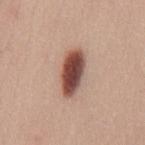No biopsy was performed on this lesion — it was imaged during a full skin examination and was not determined to be concerning. A lesion tile, about 15 mm wide, cut from a 3D total-body photograph. The lesion is located on the mid back. A female subject in their mid-20s.